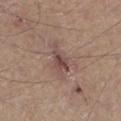The lesion was photographed on a routine skin check and not biopsied; there is no pathology result.
Captured under white-light illumination.
A 15 mm close-up extracted from a 3D total-body photography capture.
Located on the left lower leg.
A male patient, approximately 40 years of age.